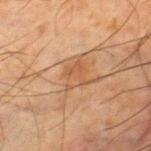Q: Was a biopsy performed?
A: catalogued during a skin exam; not biopsied
Q: What are the patient's age and sex?
A: male, approximately 65 years of age
Q: Lesion location?
A: the right thigh
Q: What is the imaging modality?
A: ~15 mm tile from a whole-body skin photo
Q: What did automated image analysis measure?
A: an average lesion color of about L≈44 a*≈18 b*≈30 (CIELAB), roughly 6 lightness units darker than nearby skin, and a lesion-to-skin contrast of about 5 (normalized; higher = more distinct); a classifier nevus-likeness of about 25/100 and lesion-presence confidence of about 95/100
Q: What lighting was used for the tile?
A: cross-polarized illumination
Q: What is the lesion's diameter?
A: ≈3 mm